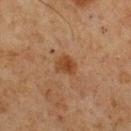{
  "biopsy_status": "not biopsied; imaged during a skin examination",
  "lesion_size": {
    "long_diameter_mm_approx": 2.5
  },
  "site": "chest",
  "image": {
    "source": "total-body photography crop",
    "field_of_view_mm": 15
  },
  "patient": {
    "sex": "male",
    "age_approx": 75
  }
}